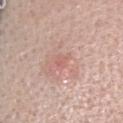Clinical impression:
The lesion was tiled from a total-body skin photograph and was not biopsied.
Acquisition and patient details:
A 15 mm close-up tile from a total-body photography series done for melanoma screening. The lesion is located on the head or neck. Longest diameter approximately 1.5 mm. A female subject approximately 45 years of age. Imaged with white-light lighting.